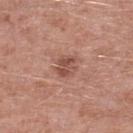The lesion is on the leg. A region of skin cropped from a whole-body photographic capture, roughly 15 mm wide. The patient is a male aged 58–62. Imaged with white-light lighting.